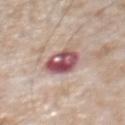<record>
<biopsy_status>not biopsied; imaged during a skin examination</biopsy_status>
<image>
  <source>total-body photography crop</source>
  <field_of_view_mm>15</field_of_view_mm>
</image>
<site>abdomen</site>
<patient>
  <sex>male</sex>
  <age_approx>65</age_approx>
</patient>
<lighting>white-light</lighting>
</record>